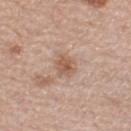The lesion was photographed on a routine skin check and not biopsied; there is no pathology result.
A female patient, aged 63–67.
The lesion is located on the right lower leg.
A lesion tile, about 15 mm wide, cut from a 3D total-body photograph.